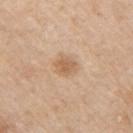Imaged during a routine full-body skin examination; the lesion was not biopsied and no histopathology is available.
The tile uses white-light illumination.
The patient is a male roughly 60 years of age.
From the right upper arm.
A region of skin cropped from a whole-body photographic capture, roughly 15 mm wide.
Measured at roughly 2.5 mm in maximum diameter.